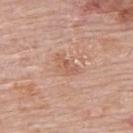workup: no biopsy performed (imaged during a skin exam)
site: the upper back
automated metrics: an area of roughly 4.5 mm², an outline eccentricity of about 0.8 (0 = round, 1 = elongated), and a symmetry-axis asymmetry near 0.4; a within-lesion color-variation index near 3/10; lesion-presence confidence of about 100/100
subject: female, approximately 65 years of age
acquisition: 15 mm crop, total-body photography